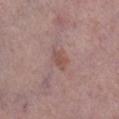Notes:
* location — the leg
* acquisition — total-body-photography crop, ~15 mm field of view
* subject — female, aged approximately 40